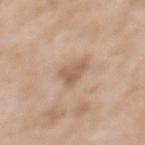Impression:
Imaged during a routine full-body skin examination; the lesion was not biopsied and no histopathology is available.